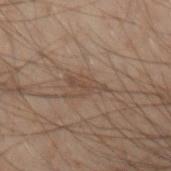  biopsy_status: not biopsied; imaged during a skin examination
  patient:
    sex: male
    age_approx: 50
  lighting: cross-polarized
  site: left arm
  image:
    source: total-body photography crop
    field_of_view_mm: 15
  automated_metrics:
    eccentricity: 0.9
    shape_asymmetry: 0.45
    nevus_likeness_0_100: 0
    lesion_detection_confidence_0_100: 85